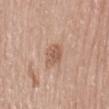Q: Is there a histopathology result?
A: no biopsy performed (imaged during a skin exam)
Q: Where on the body is the lesion?
A: the mid back
Q: What did automated image analysis measure?
A: a lesion color around L≈57 a*≈20 b*≈29 in CIELAB, about 9 CIELAB-L* units darker than the surrounding skin, and a normalized border contrast of about 6.5; a detector confidence of about 100 out of 100 that the crop contains a lesion
Q: What is the imaging modality?
A: total-body-photography crop, ~15 mm field of view
Q: Lesion size?
A: ~3 mm (longest diameter)
Q: Patient demographics?
A: female, aged approximately 70